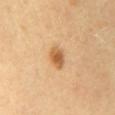Part of a total-body skin-imaging series; this lesion was reviewed on a skin check and was not flagged for biopsy.
A roughly 15 mm field-of-view crop from a total-body skin photograph.
The subject is a female aged around 60.
Captured under cross-polarized illumination.
The lesion is located on the lower back.
Longest diameter approximately 3 mm.